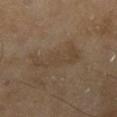Q: Was this lesion biopsied?
A: catalogued during a skin exam; not biopsied
Q: What did automated image analysis measure?
A: a shape eccentricity near 0.95 and two-axis asymmetry of about 0.5
Q: Who is the patient?
A: male, roughly 65 years of age
Q: What is the imaging modality?
A: ~15 mm crop, total-body skin-cancer survey
Q: Lesion location?
A: the right lower leg
Q: What is the lesion's diameter?
A: about 6 mm
Q: How was the tile lit?
A: cross-polarized